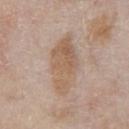Findings:
* follow-up: total-body-photography surveillance lesion; no biopsy
* image-analysis metrics: an average lesion color of about L≈59 a*≈16 b*≈30 (CIELAB) and a lesion-to-skin contrast of about 6.5 (normalized; higher = more distinct); a color-variation rating of about 4.5/10 and a peripheral color-asymmetry measure near 1.5
* acquisition: 15 mm crop, total-body photography
* location: the chest
* diameter: ≈7.5 mm
* subject: male, about 65 years old
* tile lighting: white-light illumination